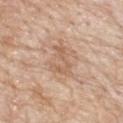{
  "patient": {
    "sex": "male",
    "age_approx": "85+"
  },
  "image": {
    "source": "total-body photography crop",
    "field_of_view_mm": 15
  },
  "lesion_size": {
    "long_diameter_mm_approx": 4.5
  },
  "site": "back",
  "lighting": "white-light",
  "automated_metrics": {
    "color_variation_0_10": 1.5,
    "peripheral_color_asymmetry": 0.5
  }
}